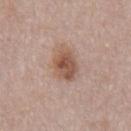Recorded during total-body skin imaging; not selected for excision or biopsy. A male patient, roughly 55 years of age. About 4 mm across. From the chest. This image is a 15 mm lesion crop taken from a total-body photograph.